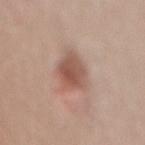Findings:
– follow-up · total-body-photography surveillance lesion; no biopsy
– image-analysis metrics · internal color variation of about 4.5 on a 0–10 scale; an automated nevus-likeness rating near 85 out of 100 and a lesion-detection confidence of about 100/100
– site · the lower back
– subject · male, about 45 years old
– illumination · white-light illumination
– size · ~4 mm (longest diameter)
– image · ~15 mm crop, total-body skin-cancer survey